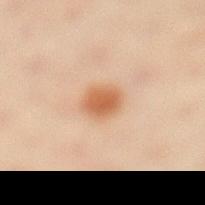Background: A female patient, roughly 45 years of age. A 15 mm close-up tile from a total-body photography series done for melanoma screening. Approximately 3 mm at its widest. The lesion is on the left lower leg. The tile uses cross-polarized illumination.